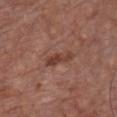Impression:
Recorded during total-body skin imaging; not selected for excision or biopsy.
Image and clinical context:
The lesion is on the chest. Automated image analysis of the tile measured a footprint of about 5 mm², a shape eccentricity near 0.95, and two-axis asymmetry of about 0.4. The analysis additionally found a lesion color around L≈41 a*≈22 b*≈27 in CIELAB, roughly 9 lightness units darker than nearby skin, and a normalized lesion–skin contrast near 7.5. And it measured border irregularity of about 4.5 on a 0–10 scale and a peripheral color-asymmetry measure near 0.5. The software also gave lesion-presence confidence of about 100/100. Cropped from a total-body skin-imaging series; the visible field is about 15 mm. A male patient in their mid- to late 60s. Imaged with white-light lighting. Approximately 4 mm at its widest.